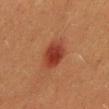notes: catalogued during a skin exam; not biopsied | lesion size: ~4 mm (longest diameter) | acquisition: total-body-photography crop, ~15 mm field of view | lighting: cross-polarized | subject: male, roughly 40 years of age | location: the mid back.